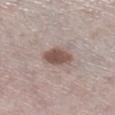Clinical impression: The lesion was photographed on a routine skin check and not biopsied; there is no pathology result. Context: A region of skin cropped from a whole-body photographic capture, roughly 15 mm wide. From the right lower leg. Approximately 4 mm at its widest. The total-body-photography lesion software estimated an area of roughly 7.5 mm², a shape eccentricity near 0.8, and a symmetry-axis asymmetry near 0.2. It also reported an average lesion color of about L≈52 a*≈16 b*≈22 (CIELAB), about 13 CIELAB-L* units darker than the surrounding skin, and a normalized lesion–skin contrast near 9.5. It also reported border irregularity of about 2 on a 0–10 scale and peripheral color asymmetry of about 1. A male subject in their 60s. Imaged with white-light lighting.